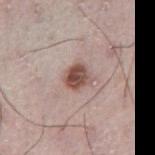notes — no biopsy performed (imaged during a skin exam) | body site — the front of the torso | image source — total-body-photography crop, ~15 mm field of view | TBP lesion metrics — a lesion color around L≈50 a*≈20 b*≈23 in CIELAB and a lesion-to-skin contrast of about 10.5 (normalized; higher = more distinct); border irregularity of about 2 on a 0–10 scale, a within-lesion color-variation index near 6/10, and a peripheral color-asymmetry measure near 2; an automated nevus-likeness rating near 90 out of 100 and a lesion-detection confidence of about 100/100 | patient — male, approximately 75 years of age.